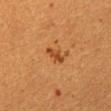workup: imaged on a skin check; not biopsied | illumination: cross-polarized illumination | patient: female, about 55 years old | lesion diameter: ~3 mm (longest diameter) | location: the abdomen | acquisition: 15 mm crop, total-body photography | automated metrics: a border-irregularity index near 3/10, a within-lesion color-variation index near 0.5/10, and peripheral color asymmetry of about 0.